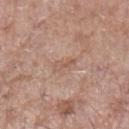Impression: Captured during whole-body skin photography for melanoma surveillance; the lesion was not biopsied.